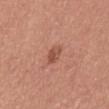Q: Who is the patient?
A: female, roughly 50 years of age
Q: Lesion location?
A: the mid back
Q: How was the tile lit?
A: white-light
Q: What kind of image is this?
A: 15 mm crop, total-body photography